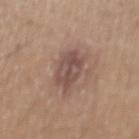follow-up = imaged on a skin check; not biopsied
patient = male, aged 18–22
body site = the mid back
imaging modality = total-body-photography crop, ~15 mm field of view